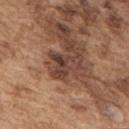follow-up — total-body-photography surveillance lesion; no biopsy | patient — male, approximately 75 years of age | tile lighting — white-light illumination | imaging modality — ~15 mm tile from a whole-body skin photo | location — the upper back | diameter — ~4 mm (longest diameter).